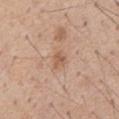workup: imaged on a skin check; not biopsied
acquisition: 15 mm crop, total-body photography
lighting: white-light illumination
anatomic site: the chest
subject: male, in their 60s